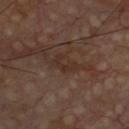biopsy status: total-body-photography surveillance lesion; no biopsy
subject: male, aged 58–62
body site: the chest
image: ~15 mm tile from a whole-body skin photo
lesion diameter: ~3 mm (longest diameter)
tile lighting: cross-polarized illumination
automated lesion analysis: a lesion–skin lightness drop of about 5; a border-irregularity index near 6.5/10, a within-lesion color-variation index near 0/10, and radial color variation of about 0